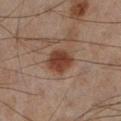Assessment: Part of a total-body skin-imaging series; this lesion was reviewed on a skin check and was not flagged for biopsy. Context: Longest diameter approximately 4 mm. A 15 mm crop from a total-body photograph taken for skin-cancer surveillance. A male subject aged 43–47. Captured under cross-polarized illumination. On the left lower leg.